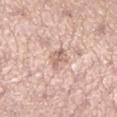Findings:
* workup — catalogued during a skin exam; not biopsied
* automated metrics — a footprint of about 4.5 mm², an eccentricity of roughly 0.7, and a shape-asymmetry score of about 0.3 (0 = symmetric); border irregularity of about 3.5 on a 0–10 scale, internal color variation of about 3 on a 0–10 scale, and radial color variation of about 1.5; an automated nevus-likeness rating near 0 out of 100 and a lesion-detection confidence of about 70/100
* patient — female, aged 33 to 37
* size — ~3 mm (longest diameter)
* illumination — white-light
* location — the left lower leg
* acquisition — ~15 mm tile from a whole-body skin photo